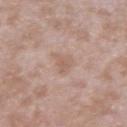<lesion>
<biopsy_status>not biopsied; imaged during a skin examination</biopsy_status>
<lesion_size>
  <long_diameter_mm_approx>3.0</long_diameter_mm_approx>
</lesion_size>
<image>
  <source>total-body photography crop</source>
  <field_of_view_mm>15</field_of_view_mm>
</image>
<patient>
  <sex>male</sex>
  <age_approx>45</age_approx>
</patient>
<site>left upper arm</site>
<automated_metrics>
  <area_mm2_approx>4.0</area_mm2_approx>
  <eccentricity>0.75</eccentricity>
  <shape_asymmetry>0.45</shape_asymmetry>
  <cielab_L>59</cielab_L>
  <cielab_a>18</cielab_a>
  <cielab_b>26</cielab_b>
  <vs_skin_darker_L>7.0</vs_skin_darker_L>
  <vs_skin_contrast_norm>5.0</vs_skin_contrast_norm>
  <color_variation_0_10>1.5</color_variation_0_10>
  <nevus_likeness_0_100>0</nevus_likeness_0_100>
  <lesion_detection_confidence_0_100>100</lesion_detection_confidence_0_100>
</automated_metrics>
<lighting>white-light</lighting>
</lesion>